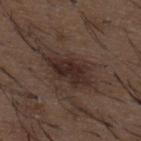Q: Was this lesion biopsied?
A: no biopsy performed (imaged during a skin exam)
Q: What did automated image analysis measure?
A: a mean CIELAB color near L≈28 a*≈14 b*≈19, a lesion–skin lightness drop of about 8, and a normalized lesion–skin contrast near 8.5; a classifier nevus-likeness of about 30/100 and a lesion-detection confidence of about 100/100
Q: What is the anatomic site?
A: the upper back
Q: Lesion size?
A: ~6 mm (longest diameter)
Q: How was the tile lit?
A: white-light
Q: What are the patient's age and sex?
A: male, in their 50s
Q: What is the imaging modality?
A: ~15 mm tile from a whole-body skin photo